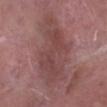Clinical impression:
Captured during whole-body skin photography for melanoma surveillance; the lesion was not biopsied.
Image and clinical context:
From the left lower leg. A 15 mm crop from a total-body photograph taken for skin-cancer surveillance. The patient is a male approximately 40 years of age.